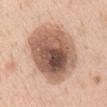Assessment: Part of a total-body skin-imaging series; this lesion was reviewed on a skin check and was not flagged for biopsy. Clinical summary: A female subject aged around 50. The lesion's longest dimension is about 10 mm. Located on the mid back. A 15 mm close-up extracted from a 3D total-body photography capture. Imaged with white-light lighting.